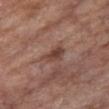workup: no biopsy performed (imaged during a skin exam) | imaging modality: total-body-photography crop, ~15 mm field of view | body site: the chest | diameter: ≈3.5 mm | subject: female, about 75 years old.